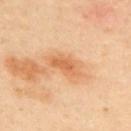{"biopsy_status": "not biopsied; imaged during a skin examination", "lesion_size": {"long_diameter_mm_approx": 4.0}, "site": "upper back", "image": {"source": "total-body photography crop", "field_of_view_mm": 15}, "lighting": "cross-polarized", "patient": {"sex": "male", "age_approx": 50}}